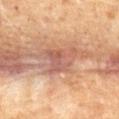| key | value |
|---|---|
| notes | no biopsy performed (imaged during a skin exam) |
| image-analysis metrics | a border-irregularity rating of about 5.5/10, internal color variation of about 5 on a 0–10 scale, and radial color variation of about 2 |
| location | the chest |
| acquisition | ~15 mm tile from a whole-body skin photo |
| illumination | cross-polarized illumination |
| lesion diameter | ~4.5 mm (longest diameter) |
| patient | male, aged 63–67 |